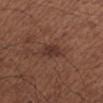Notes:
– workup · total-body-photography surveillance lesion; no biopsy
– subject · male, aged approximately 65
– image-analysis metrics · a footprint of about 4.5 mm², an outline eccentricity of about 0.6 (0 = round, 1 = elongated), and a symmetry-axis asymmetry near 0.35; a border-irregularity index near 3/10, internal color variation of about 2 on a 0–10 scale, and a peripheral color-asymmetry measure near 0.5; an automated nevus-likeness rating near 45 out of 100 and lesion-presence confidence of about 100/100
– diameter · ≈2.5 mm
– image source · total-body-photography crop, ~15 mm field of view
– location · the right forearm